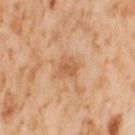{"biopsy_status": "not biopsied; imaged during a skin examination", "image": {"source": "total-body photography crop", "field_of_view_mm": 15}, "site": "right thigh", "automated_metrics": {"cielab_L": 60, "cielab_a": 23, "cielab_b": 38, "vs_skin_darker_L": 9.0, "nevus_likeness_0_100": 0, "lesion_detection_confidence_0_100": 100}, "lesion_size": {"long_diameter_mm_approx": 2.5}, "lighting": "cross-polarized", "patient": {"sex": "female", "age_approx": 55}}